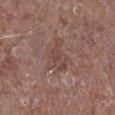notes: total-body-photography surveillance lesion; no biopsy
location: the leg
patient: male, in their mid-40s
size: ≈4 mm
illumination: white-light illumination
image source: ~15 mm tile from a whole-body skin photo
automated lesion analysis: a lesion area of about 9 mm², an eccentricity of roughly 0.65, and a shape-asymmetry score of about 0.45 (0 = symmetric); a mean CIELAB color near L≈45 a*≈19 b*≈22, roughly 6 lightness units darker than nearby skin, and a normalized lesion–skin contrast near 5; a border-irregularity rating of about 5.5/10, a within-lesion color-variation index near 2.5/10, and peripheral color asymmetry of about 1; an automated nevus-likeness rating near 0 out of 100